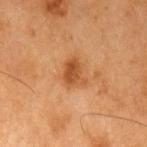{"biopsy_status": "not biopsied; imaged during a skin examination", "lesion_size": {"long_diameter_mm_approx": 3.0}, "lighting": "cross-polarized", "image": {"source": "total-body photography crop", "field_of_view_mm": 15}, "patient": {"sex": "male", "age_approx": 60}, "site": "arm"}